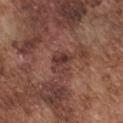<record>
  <biopsy_status>not biopsied; imaged during a skin examination</biopsy_status>
  <image>
    <source>total-body photography crop</source>
    <field_of_view_mm>15</field_of_view_mm>
  </image>
  <site>chest</site>
  <lesion_size>
    <long_diameter_mm_approx>3.0</long_diameter_mm_approx>
  </lesion_size>
  <lighting>white-light</lighting>
  <automated_metrics>
    <nevus_likeness_0_100>0</nevus_likeness_0_100>
  </automated_metrics>
  <patient>
    <sex>male</sex>
    <age_approx>75</age_approx>
  </patient>
</record>